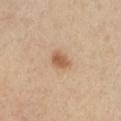Clinical impression: Part of a total-body skin-imaging series; this lesion was reviewed on a skin check and was not flagged for biopsy. Background: A roughly 15 mm field-of-view crop from a total-body skin photograph. The recorded lesion diameter is about 2.5 mm. The patient is a female in their mid-60s. Located on the chest.